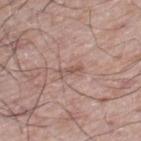– follow-up: total-body-photography surveillance lesion; no biopsy
– lighting: white-light illumination
– site: the left thigh
– subject: male, aged 58 to 62
– image: ~15 mm crop, total-body skin-cancer survey
– size: ≈2.5 mm